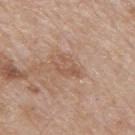notes = imaged on a skin check; not biopsied
tile lighting = white-light
anatomic site = the mid back
image-analysis metrics = a mean CIELAB color near L≈55 a*≈19 b*≈30, a lesion–skin lightness drop of about 7, and a normalized border contrast of about 5; an automated nevus-likeness rating near 0 out of 100 and a lesion-detection confidence of about 100/100
image source = ~15 mm tile from a whole-body skin photo
subject = male, in their mid- to late 60s